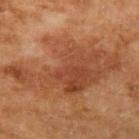A lesion tile, about 15 mm wide, cut from a 3D total-body photograph. Longest diameter approximately 13.5 mm. The tile uses cross-polarized illumination. On the left upper arm. A male subject about 55 years old.